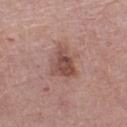notes = no biopsy performed (imaged during a skin exam) | image source = ~15 mm crop, total-body skin-cancer survey | patient = female, roughly 65 years of age | lighting = white-light illumination | anatomic site = the left thigh | automated metrics = an average lesion color of about L≈49 a*≈21 b*≈24 (CIELAB) and about 11 CIELAB-L* units darker than the surrounding skin; a border-irregularity rating of about 3.5/10, a within-lesion color-variation index near 4.5/10, and peripheral color asymmetry of about 1.5 | diameter = ~4 mm (longest diameter).